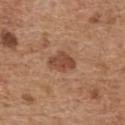Clinical impression: Imaged during a routine full-body skin examination; the lesion was not biopsied and no histopathology is available. Acquisition and patient details: A lesion tile, about 15 mm wide, cut from a 3D total-body photograph. The lesion is on the upper back. Automated image analysis of the tile measured a border-irregularity index near 2/10 and radial color variation of about 0.5. A male patient aged 68 to 72. About 3.5 mm across. Imaged with white-light lighting.